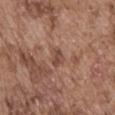{
  "biopsy_status": "not biopsied; imaged during a skin examination",
  "site": "abdomen",
  "lesion_size": {
    "long_diameter_mm_approx": 2.5
  },
  "image": {
    "source": "total-body photography crop",
    "field_of_view_mm": 15
  },
  "automated_metrics": {
    "area_mm2_approx": 4.0,
    "eccentricity": 0.8,
    "shape_asymmetry": 0.25,
    "nevus_likeness_0_100": 0,
    "lesion_detection_confidence_0_100": 85
  },
  "lighting": "white-light",
  "patient": {
    "sex": "male",
    "age_approx": 75
  }
}